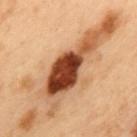No biopsy was performed on this lesion — it was imaged during a full skin examination and was not determined to be concerning.